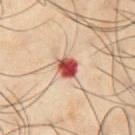This lesion was catalogued during total-body skin photography and was not selected for biopsy. A male patient, aged approximately 50. A 15 mm close-up tile from a total-body photography series done for melanoma screening. Located on the abdomen.